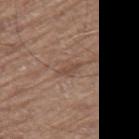Impression: Part of a total-body skin-imaging series; this lesion was reviewed on a skin check and was not flagged for biopsy. Clinical summary: Located on the right thigh. This is a white-light tile. A 15 mm close-up extracted from a 3D total-body photography capture. Approximately 3 mm at its widest. A male subject aged around 70.